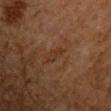Impression: The lesion was photographed on a routine skin check and not biopsied; there is no pathology result. Image and clinical context: A male patient, approximately 65 years of age. Automated tile analysis of the lesion measured a lesion area of about 3 mm² and an outline eccentricity of about 0.95 (0 = round, 1 = elongated). It also reported a lesion color around L≈26 a*≈16 b*≈26 in CIELAB, about 4 CIELAB-L* units darker than the surrounding skin, and a normalized border contrast of about 6. The analysis additionally found border irregularity of about 4 on a 0–10 scale, internal color variation of about 0 on a 0–10 scale, and a peripheral color-asymmetry measure near 0. It also reported a classifier nevus-likeness of about 0/100 and lesion-presence confidence of about 95/100. The lesion is on the chest. Approximately 3 mm at its widest. A roughly 15 mm field-of-view crop from a total-body skin photograph. This is a cross-polarized tile.